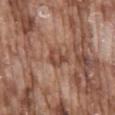image: 15 mm crop, total-body photography
site: the mid back
image-analysis metrics: a footprint of about 5 mm² and an outline eccentricity of about 0.65 (0 = round, 1 = elongated); a lesion color around L≈47 a*≈22 b*≈28 in CIELAB, a lesion–skin lightness drop of about 9, and a lesion-to-skin contrast of about 6.5 (normalized; higher = more distinct); border irregularity of about 3 on a 0–10 scale, a within-lesion color-variation index near 4.5/10, and radial color variation of about 1.5
subject: male, roughly 75 years of age
lesion diameter: ~3 mm (longest diameter)
illumination: white-light